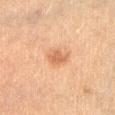Assessment: The lesion was tiled from a total-body skin photograph and was not biopsied. Image and clinical context: Automated image analysis of the tile measured an average lesion color of about L≈58 a*≈22 b*≈35 (CIELAB), roughly 9 lightness units darker than nearby skin, and a lesion-to-skin contrast of about 6.5 (normalized; higher = more distinct). It also reported a lesion-detection confidence of about 100/100. A close-up tile cropped from a whole-body skin photograph, about 15 mm across. The subject is a female about 30 years old. The tile uses cross-polarized illumination. Located on the abdomen. The recorded lesion diameter is about 3 mm.